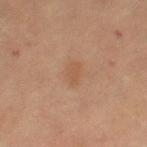Part of a total-body skin-imaging series; this lesion was reviewed on a skin check and was not flagged for biopsy. About 2.5 mm across. This image is a 15 mm lesion crop taken from a total-body photograph. This is a cross-polarized tile. The lesion is on the left thigh. The patient is a female aged 68–72.